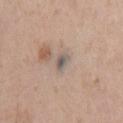Assessment: Recorded during total-body skin imaging; not selected for excision or biopsy. Background: Cropped from a total-body skin-imaging series; the visible field is about 15 mm. The lesion is on the chest. About 2.5 mm across. The patient is a male roughly 55 years of age.